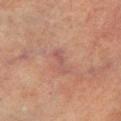workup: total-body-photography surveillance lesion; no biopsy
subject: male, about 75 years old
anatomic site: the leg
image-analysis metrics: an average lesion color of about L≈43 a*≈19 b*≈21 (CIELAB) and a lesion-to-skin contrast of about 5.5 (normalized; higher = more distinct); a border-irregularity index near 4.5/10, a color-variation rating of about 0/10, and a peripheral color-asymmetry measure near 0
tile lighting: cross-polarized illumination
lesion size: ≈3 mm
acquisition: 15 mm crop, total-body photography